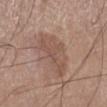  biopsy_status: not biopsied; imaged during a skin examination
  lesion_size:
    long_diameter_mm_approx: 6.0
  site: leg
  patient:
    sex: male
    age_approx: 60
  lighting: white-light
  image:
    source: total-body photography crop
    field_of_view_mm: 15